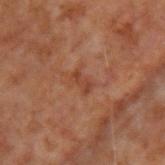Impression:
Captured during whole-body skin photography for melanoma surveillance; the lesion was not biopsied.
Image and clinical context:
Located on the upper back. A 15 mm crop from a total-body photograph taken for skin-cancer surveillance. Imaged with cross-polarized lighting. A male subject, aged 68–72. Automated tile analysis of the lesion measured an area of roughly 3 mm². The software also gave a lesion color around L≈32 a*≈19 b*≈25 in CIELAB, a lesion–skin lightness drop of about 5, and a lesion-to-skin contrast of about 5.5 (normalized; higher = more distinct). Approximately 2.5 mm at its widest.